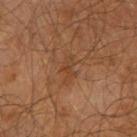- notes · no biopsy performed (imaged during a skin exam)
- body site · the right upper arm
- illumination · cross-polarized
- image source · ~15 mm crop, total-body skin-cancer survey
- subject · male, in their mid- to late 60s
- automated metrics · an average lesion color of about L≈39 a*≈20 b*≈31 (CIELAB), about 5 CIELAB-L* units darker than the surrounding skin, and a normalized lesion–skin contrast near 5; a classifier nevus-likeness of about 0/100